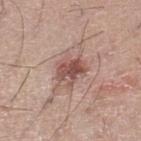Background: The subject is a male in their 20s. The total-body-photography lesion software estimated a lesion area of about 7 mm². The analysis additionally found a mean CIELAB color near L≈51 a*≈22 b*≈23, a lesion–skin lightness drop of about 12, and a lesion-to-skin contrast of about 8.5 (normalized; higher = more distinct). The analysis additionally found border irregularity of about 3.5 on a 0–10 scale, a color-variation rating of about 4.5/10, and a peripheral color-asymmetry measure near 1.5. It also reported lesion-presence confidence of about 100/100. The tile uses white-light illumination. The lesion is on the leg. A lesion tile, about 15 mm wide, cut from a 3D total-body photograph. About 3.5 mm across.